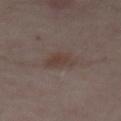Impression:
The lesion was tiled from a total-body skin photograph and was not biopsied.
Context:
A 15 mm close-up extracted from a 3D total-body photography capture. Approximately 3 mm at its widest. From the abdomen. The patient is aged 53 to 57. Imaged with cross-polarized lighting.